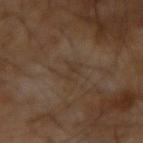Background: The total-body-photography lesion software estimated a lesion color around L≈33 a*≈13 b*≈24 in CIELAB, a lesion–skin lightness drop of about 4, and a normalized border contrast of about 4.5. Cropped from a whole-body photographic skin survey; the tile spans about 15 mm. Longest diameter approximately 2.5 mm. A male patient, roughly 65 years of age.